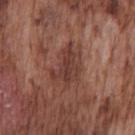notes = imaged on a skin check; not biopsied
subject = male, aged approximately 75
TBP lesion metrics = a mean CIELAB color near L≈38 a*≈22 b*≈24, roughly 9 lightness units darker than nearby skin, and a normalized lesion–skin contrast near 7.5; an automated nevus-likeness rating near 0 out of 100 and a detector confidence of about 95 out of 100 that the crop contains a lesion
illumination = white-light illumination
acquisition = ~15 mm crop, total-body skin-cancer survey
anatomic site = the chest
diameter = about 4.5 mm